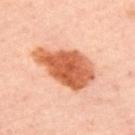biopsy status=no biopsy performed (imaged during a skin exam); anatomic site=the upper back; subject=about 55 years old; acquisition=15 mm crop, total-body photography; lesion diameter=about 7.5 mm; tile lighting=cross-polarized illumination.